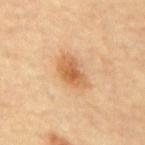Findings:
• workup · imaged on a skin check; not biopsied
• image source · ~15 mm tile from a whole-body skin photo
• diameter · about 4 mm
• patient · male, roughly 85 years of age or older
• illumination · cross-polarized
• site · the mid back